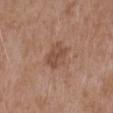| field | value |
|---|---|
| patient | male, about 50 years old |
| tile lighting | white-light |
| diameter | about 3.5 mm |
| image-analysis metrics | about 8 CIELAB-L* units darker than the surrounding skin; a border-irregularity index near 2/10, a within-lesion color-variation index near 2.5/10, and a peripheral color-asymmetry measure near 1; a classifier nevus-likeness of about 15/100 |
| site | the left upper arm |
| acquisition | ~15 mm tile from a whole-body skin photo |